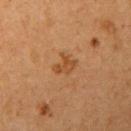notes: no biopsy performed (imaged during a skin exam) | image source: total-body-photography crop, ~15 mm field of view | location: the right upper arm | subject: female, approximately 40 years of age.